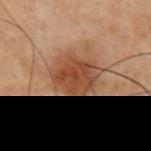Q: Is there a histopathology result?
A: total-body-photography surveillance lesion; no biopsy
Q: Patient demographics?
A: male, in their 70s
Q: How was the tile lit?
A: cross-polarized illumination
Q: What is the anatomic site?
A: the chest
Q: How was this image acquired?
A: ~15 mm crop, total-body skin-cancer survey